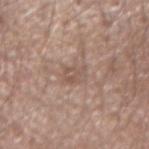The lesion is located on the left forearm. Cropped from a total-body skin-imaging series; the visible field is about 15 mm. Measured at roughly 2.5 mm in maximum diameter. The total-body-photography lesion software estimated a footprint of about 5.5 mm² and two-axis asymmetry of about 0.2. And it measured a border-irregularity rating of about 2.5/10 and a within-lesion color-variation index near 3.5/10. The software also gave a nevus-likeness score of about 0/100 and lesion-presence confidence of about 95/100. The patient is a male in their mid- to late 50s.